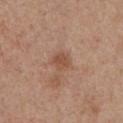Part of a total-body skin-imaging series; this lesion was reviewed on a skin check and was not flagged for biopsy. The lesion is located on the chest. About 2.5 mm across. A male subject aged approximately 55. The total-body-photography lesion software estimated an area of roughly 4.5 mm², a shape eccentricity near 0.55, and a shape-asymmetry score of about 0.25 (0 = symmetric). It also reported a border-irregularity rating of about 2/10 and a peripheral color-asymmetry measure near 1. And it measured a nevus-likeness score of about 5/100 and a detector confidence of about 100 out of 100 that the crop contains a lesion. Imaged with white-light lighting. This image is a 15 mm lesion crop taken from a total-body photograph.